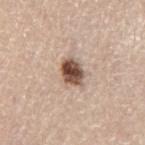<record>
<biopsy_status>not biopsied; imaged during a skin examination</biopsy_status>
<lighting>white-light</lighting>
<patient>
  <sex>female</sex>
  <age_approx>60</age_approx>
</patient>
<lesion_size>
  <long_diameter_mm_approx>3.5</long_diameter_mm_approx>
</lesion_size>
<site>leg</site>
<image>
  <source>total-body photography crop</source>
  <field_of_view_mm>15</field_of_view_mm>
</image>
</record>